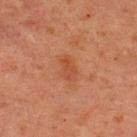The lesion was tiled from a total-body skin photograph and was not biopsied.
Located on the upper back.
A male patient, about 60 years old.
A lesion tile, about 15 mm wide, cut from a 3D total-body photograph.
Measured at roughly 3 mm in maximum diameter.